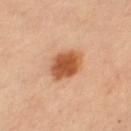This lesion was catalogued during total-body skin photography and was not selected for biopsy.
This image is a 15 mm lesion crop taken from a total-body photograph.
Imaged with cross-polarized lighting.
A female subject aged 68 to 72.
Automated image analysis of the tile measured a footprint of about 11 mm², an eccentricity of roughly 0.35, and two-axis asymmetry of about 0.15. It also reported a mean CIELAB color near L≈57 a*≈27 b*≈39, a lesion–skin lightness drop of about 15, and a normalized lesion–skin contrast near 10. The analysis additionally found border irregularity of about 1.5 on a 0–10 scale, a color-variation rating of about 4.5/10, and peripheral color asymmetry of about 1. It also reported an automated nevus-likeness rating near 100 out of 100 and a lesion-detection confidence of about 100/100.
On the right thigh.
About 3.5 mm across.